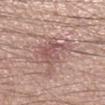Impression:
The lesion was tiled from a total-body skin photograph and was not biopsied.
Clinical summary:
The patient is a male aged 58–62. Imaged with white-light lighting. The lesion-visualizer software estimated a lesion area of about 15 mm², an outline eccentricity of about 0.7 (0 = round, 1 = elongated), and a shape-asymmetry score of about 0.45 (0 = symmetric). It also reported a lesion color around L≈55 a*≈20 b*≈22 in CIELAB, a lesion–skin lightness drop of about 9, and a lesion-to-skin contrast of about 6 (normalized; higher = more distinct). And it measured a border-irregularity rating of about 6/10, a within-lesion color-variation index near 5/10, and peripheral color asymmetry of about 1.5. From the right lower leg. A 15 mm crop from a total-body photograph taken for skin-cancer surveillance.